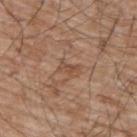| key | value |
|---|---|
| workup | imaged on a skin check; not biopsied |
| image source | 15 mm crop, total-body photography |
| subject | male, approximately 65 years of age |
| lighting | white-light illumination |
| automated lesion analysis | a footprint of about 3 mm², an outline eccentricity of about 0.85 (0 = round, 1 = elongated), and a shape-asymmetry score of about 0.45 (0 = symmetric); lesion-presence confidence of about 90/100 |
| diameter | ≈2.5 mm |
| location | the upper back |